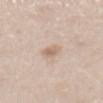The lesion was tiled from a total-body skin photograph and was not biopsied. A male patient, aged 38–42. Located on the abdomen. Cropped from a whole-body photographic skin survey; the tile spans about 15 mm. This is a white-light tile.